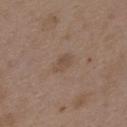- notes · catalogued during a skin exam; not biopsied
- location · the upper back
- patient · female, approximately 35 years of age
- lesion diameter · ~2.5 mm (longest diameter)
- imaging modality · ~15 mm tile from a whole-body skin photo
- lighting · white-light